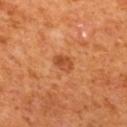Findings:
• follow-up — catalogued during a skin exam; not biopsied
• image source — ~15 mm crop, total-body skin-cancer survey
• illumination — cross-polarized illumination
• subject — male, in their mid-60s
• body site — the mid back